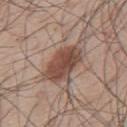The lesion was tiled from a total-body skin photograph and was not biopsied. The patient is a male in their mid-50s. The tile uses white-light illumination. The lesion's longest dimension is about 5 mm. The lesion is on the upper back. Cropped from a whole-body photographic skin survey; the tile spans about 15 mm.